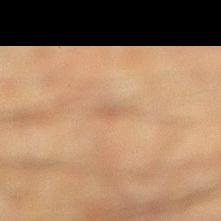Clinical summary:
The subject is a male aged around 50. A roughly 15 mm field-of-view crop from a total-body skin photograph. The lesion is located on the right lower leg.